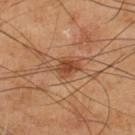biopsy status: total-body-photography surveillance lesion; no biopsy | tile lighting: cross-polarized | anatomic site: the left lower leg | patient: male, aged 63–67 | automated metrics: a within-lesion color-variation index near 2.5/10 and peripheral color asymmetry of about 1 | imaging modality: 15 mm crop, total-body photography.